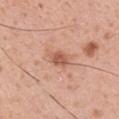Clinical impression:
The lesion was tiled from a total-body skin photograph and was not biopsied.
Clinical summary:
The tile uses white-light illumination. Cropped from a total-body skin-imaging series; the visible field is about 15 mm. A male subject about 30 years old. The recorded lesion diameter is about 3 mm. On the arm.